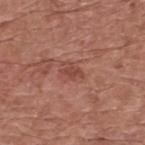The lesion was tiled from a total-body skin photograph and was not biopsied. From the upper back. A lesion tile, about 15 mm wide, cut from a 3D total-body photograph. A male subject, in their mid- to late 70s.